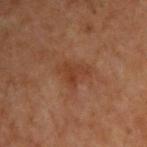Findings:
- biopsy status — imaged on a skin check; not biopsied
- acquisition — total-body-photography crop, ~15 mm field of view
- site — the upper back
- patient — male, in their 70s